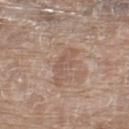Clinical impression: No biopsy was performed on this lesion — it was imaged during a full skin examination and was not determined to be concerning. Context: On the right thigh. This is a white-light tile. A female subject in their mid-70s. Cropped from a total-body skin-imaging series; the visible field is about 15 mm. The total-body-photography lesion software estimated a lesion area of about 6.5 mm², an eccentricity of roughly 0.95, and a symmetry-axis asymmetry near 0.5. It also reported an average lesion color of about L≈55 a*≈17 b*≈26 (CIELAB).